From the mid back · the patient is a female about 30 years old · a 15 mm close-up tile from a total-body photography series done for melanoma screening:
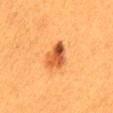Acquisition and patient details: Captured under cross-polarized illumination. Conclusion: Histopathology of the biopsied lesion showed an atypical melanocytic neoplasm — a borderline lesion of uncertain malignant potential.